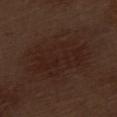Assessment: Part of a total-body skin-imaging series; this lesion was reviewed on a skin check and was not flagged for biopsy. Context: Measured at roughly 8 mm in maximum diameter. This is a white-light tile. On the left thigh. Cropped from a total-body skin-imaging series; the visible field is about 15 mm. The subject is a male aged 68–72.